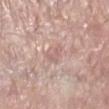Part of a total-body skin-imaging series; this lesion was reviewed on a skin check and was not flagged for biopsy. The subject is a female about 60 years old. A region of skin cropped from a whole-body photographic capture, roughly 15 mm wide. The recorded lesion diameter is about 2.5 mm. Automated image analysis of the tile measured a lesion area of about 2.5 mm², an outline eccentricity of about 0.9 (0 = round, 1 = elongated), and two-axis asymmetry of about 0.4. The software also gave a lesion color around L≈61 a*≈20 b*≈22 in CIELAB, roughly 7 lightness units darker than nearby skin, and a normalized border contrast of about 5. It also reported border irregularity of about 4.5 on a 0–10 scale and internal color variation of about 0 on a 0–10 scale. From the left lower leg.